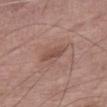Q: Who is the patient?
A: female, in their mid- to late 70s
Q: Lesion location?
A: the right thigh
Q: Automated lesion metrics?
A: a footprint of about 4.5 mm², an eccentricity of roughly 0.85, and a shape-asymmetry score of about 0.2 (0 = symmetric); a border-irregularity rating of about 2.5/10 and internal color variation of about 1.5 on a 0–10 scale
Q: Illumination type?
A: white-light illumination
Q: How large is the lesion?
A: about 3 mm
Q: What is the imaging modality?
A: total-body-photography crop, ~15 mm field of view